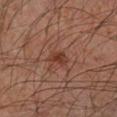Impression: Part of a total-body skin-imaging series; this lesion was reviewed on a skin check and was not flagged for biopsy. Background: A 15 mm close-up tile from a total-body photography series done for melanoma screening. Located on the left forearm. A male patient approximately 45 years of age. The lesion's longest dimension is about 2.5 mm. Captured under cross-polarized illumination.